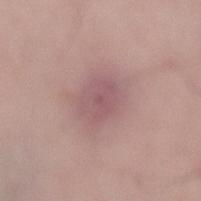Case summary:
* biopsy status: catalogued during a skin exam; not biopsied
* subject: male, approximately 50 years of age
* lighting: white-light illumination
* automated metrics: an average lesion color of about L≈55 a*≈21 b*≈17 (CIELAB), about 8 CIELAB-L* units darker than the surrounding skin, and a normalized border contrast of about 6; a color-variation rating of about 3/10 and a peripheral color-asymmetry measure near 1; an automated nevus-likeness rating near 0 out of 100 and lesion-presence confidence of about 95/100
* imaging modality: ~15 mm tile from a whole-body skin photo
* anatomic site: the lower back